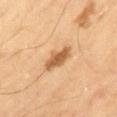Impression: Part of a total-body skin-imaging series; this lesion was reviewed on a skin check and was not flagged for biopsy. Clinical summary: Automated tile analysis of the lesion measured an area of roughly 6.5 mm² and a shape eccentricity near 0.9. A 15 mm crop from a total-body photograph taken for skin-cancer surveillance. The subject is a male aged 63–67.